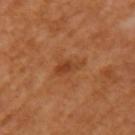  biopsy_status: not biopsied; imaged during a skin examination
  site: arm
  automated_metrics:
    eccentricity: 0.9
    vs_skin_contrast_norm: 6.5
    lesion_detection_confidence_0_100: 100
  image:
    source: total-body photography crop
    field_of_view_mm: 15
  patient:
    sex: female
    age_approx: 55